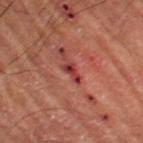Findings:
* biopsy status: catalogued during a skin exam; not biopsied
* image: total-body-photography crop, ~15 mm field of view
* patient: male, aged 53 to 57
* illumination: cross-polarized
* size: about 2.5 mm
* location: the leg
* image-analysis metrics: a lesion area of about 2.5 mm², an outline eccentricity of about 0.9 (0 = round, 1 = elongated), and a symmetry-axis asymmetry near 0.45; a lesion color around L≈34 a*≈31 b*≈23 in CIELAB; a lesion-detection confidence of about 90/100